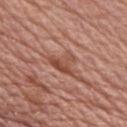{"biopsy_status": "not biopsied; imaged during a skin examination", "patient": {"sex": "male", "age_approx": 75}, "lighting": "white-light", "lesion_size": {"long_diameter_mm_approx": 4.0}, "image": {"source": "total-body photography crop", "field_of_view_mm": 15}, "automated_metrics": {"vs_skin_darker_L": 9.0, "vs_skin_contrast_norm": 7.5, "border_irregularity_0_10": 6.0, "nevus_likeness_0_100": 0}, "site": "front of the torso"}